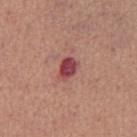| key | value |
|---|---|
| image | 15 mm crop, total-body photography |
| lesion size | about 3 mm |
| subject | male, in their mid- to late 40s |
| illumination | white-light illumination |
| image-analysis metrics | a lesion area of about 5 mm², an outline eccentricity of about 0.7 (0 = round, 1 = elongated), and a symmetry-axis asymmetry near 0.25; a border-irregularity index near 2/10, a within-lesion color-variation index near 3/10, and radial color variation of about 1; a nevus-likeness score of about 0/100 and a lesion-detection confidence of about 100/100 |
| body site | the abdomen |